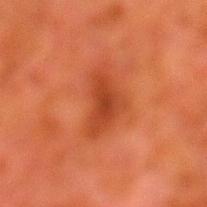{"site": "left lower leg", "patient": {"sex": "male", "age_approx": 80}, "automated_metrics": {"cielab_L": 36, "cielab_a": 29, "cielab_b": 34, "vs_skin_contrast_norm": 7.0}, "lesion_size": {"long_diameter_mm_approx": 5.0}, "image": {"source": "total-body photography crop", "field_of_view_mm": 15}, "lighting": "cross-polarized"}